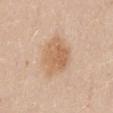Captured during whole-body skin photography for melanoma surveillance; the lesion was not biopsied.
The lesion is located on the mid back.
A 15 mm close-up extracted from a 3D total-body photography capture.
The tile uses white-light illumination.
A female subject, aged 38 to 42.